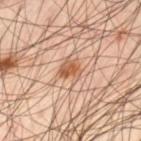{
  "biopsy_status": "not biopsied; imaged during a skin examination",
  "lighting": "cross-polarized",
  "patient": {
    "sex": "male",
    "age_approx": 45
  },
  "image": {
    "source": "total-body photography crop",
    "field_of_view_mm": 15
  },
  "lesion_size": {
    "long_diameter_mm_approx": 3.0
  },
  "site": "left thigh",
  "automated_metrics": {
    "area_mm2_approx": 6.0,
    "eccentricity": 0.45,
    "shape_asymmetry": 0.3,
    "vs_skin_darker_L": 10.0
  }
}